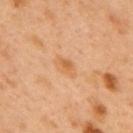biopsy status — total-body-photography surveillance lesion; no biopsy
image-analysis metrics — an area of roughly 4 mm² and two-axis asymmetry of about 0.2; internal color variation of about 2.5 on a 0–10 scale and radial color variation of about 1
lighting — cross-polarized
anatomic site — the mid back
image — 15 mm crop, total-body photography
patient — male, about 50 years old